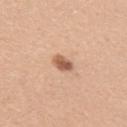Impression: Part of a total-body skin-imaging series; this lesion was reviewed on a skin check and was not flagged for biopsy. Background: Cropped from a whole-body photographic skin survey; the tile spans about 15 mm. A male subject, about 40 years old. Located on the right upper arm.